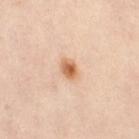biopsy status: catalogued during a skin exam; not biopsied
patient: female, aged around 40
image source: total-body-photography crop, ~15 mm field of view
location: the left leg